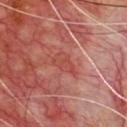workup = catalogued during a skin exam; not biopsied
acquisition = total-body-photography crop, ~15 mm field of view
lighting = cross-polarized
TBP lesion metrics = border irregularity of about 3.5 on a 0–10 scale, a color-variation rating of about 3/10, and peripheral color asymmetry of about 1
location = the chest
size = about 3 mm
subject = male, aged 68–72